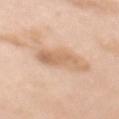Part of a total-body skin-imaging series; this lesion was reviewed on a skin check and was not flagged for biopsy. The lesion is located on the mid back. A region of skin cropped from a whole-body photographic capture, roughly 15 mm wide. The subject is a female in their mid-60s. The recorded lesion diameter is about 6 mm. Captured under white-light illumination. Automated tile analysis of the lesion measured a border-irregularity rating of about 6/10 and a within-lesion color-variation index near 3/10. The analysis additionally found a classifier nevus-likeness of about 15/100.